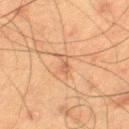biopsy status: catalogued during a skin exam; not biopsied | imaging modality: 15 mm crop, total-body photography | tile lighting: cross-polarized | lesion size: ≈3 mm | body site: the leg | patient: male, in their 60s.